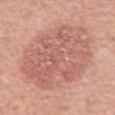The lesion was tiled from a total-body skin photograph and was not biopsied.
A lesion tile, about 15 mm wide, cut from a 3D total-body photograph.
A male patient, roughly 65 years of age.
The lesion is on the mid back.